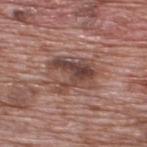notes=imaged on a skin check; not biopsied | imaging modality=~15 mm tile from a whole-body skin photo | patient=male, in their 70s | lighting=white-light | size=≈6 mm | location=the upper back.